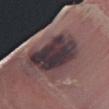Acquisition and patient details:
A male patient aged 78–82. The lesion is on the right forearm. Longest diameter approximately 7.5 mm. This is a white-light tile. An algorithmic analysis of the crop reported a lesion area of about 24 mm², a shape eccentricity near 0.85, and a shape-asymmetry score of about 0.2 (0 = symmetric). The software also gave a lesion color around L≈33 a*≈14 b*≈10 in CIELAB, about 13 CIELAB-L* units darker than the surrounding skin, and a normalized border contrast of about 13. The software also gave a border-irregularity index near 2.5/10, a within-lesion color-variation index near 6.5/10, and a peripheral color-asymmetry measure near 2. A roughly 15 mm field-of-view crop from a total-body skin photograph.